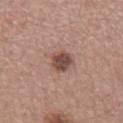No biopsy was performed on this lesion — it was imaged during a full skin examination and was not determined to be concerning.
A male patient, in their mid- to late 50s.
A 15 mm crop from a total-body photograph taken for skin-cancer surveillance.
Longest diameter approximately 3 mm.